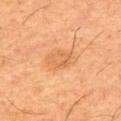Captured during whole-body skin photography for melanoma surveillance; the lesion was not biopsied.
A male subject in their 60s.
The lesion-visualizer software estimated a footprint of about 4.5 mm², an outline eccentricity of about 0.7 (0 = round, 1 = elongated), and a shape-asymmetry score of about 0.45 (0 = symmetric). And it measured a border-irregularity index near 6.5/10, a within-lesion color-variation index near 0/10, and peripheral color asymmetry of about 0. It also reported a classifier nevus-likeness of about 20/100 and lesion-presence confidence of about 100/100.
This image is a 15 mm lesion crop taken from a total-body photograph.
Longest diameter approximately 2.5 mm.
The lesion is on the back.